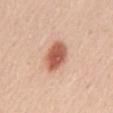Impression:
Recorded during total-body skin imaging; not selected for excision or biopsy.
Acquisition and patient details:
A roughly 15 mm field-of-view crop from a total-body skin photograph. The lesion's longest dimension is about 4.5 mm. Located on the mid back. The total-body-photography lesion software estimated an area of roughly 10 mm², an outline eccentricity of about 0.8 (0 = round, 1 = elongated), and a symmetry-axis asymmetry near 0.2. It also reported a border-irregularity rating of about 2/10 and a peripheral color-asymmetry measure near 1. A female subject, approximately 45 years of age. This is a white-light tile.